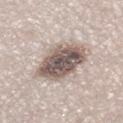| field | value |
|---|---|
| follow-up | total-body-photography surveillance lesion; no biopsy |
| patient | female, approximately 40 years of age |
| illumination | white-light |
| acquisition | total-body-photography crop, ~15 mm field of view |
| anatomic site | the right lower leg |
| diameter | ~7 mm (longest diameter) |
| automated metrics | a footprint of about 19 mm², a shape eccentricity near 0.85, and a shape-asymmetry score of about 0.2 (0 = symmetric); an average lesion color of about L≈55 a*≈13 b*≈20 (CIELAB), roughly 19 lightness units darker than nearby skin, and a normalized lesion–skin contrast near 11.5; border irregularity of about 2.5 on a 0–10 scale, a within-lesion color-variation index near 7.5/10, and peripheral color asymmetry of about 2.5 |